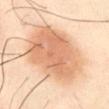* notes — no biopsy performed (imaged during a skin exam)
* subject — male, about 40 years old
* imaging modality — total-body-photography crop, ~15 mm field of view
* site — the abdomen